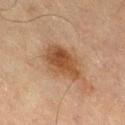notes — total-body-photography surveillance lesion; no biopsy
illumination — cross-polarized illumination
body site — the right thigh
imaging modality — ~15 mm crop, total-body skin-cancer survey
subject — male, aged approximately 70